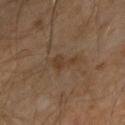follow-up — catalogued during a skin exam; not biopsied
lighting — cross-polarized
patient — male, aged 68–72
site — the right forearm
imaging modality — ~15 mm tile from a whole-body skin photo